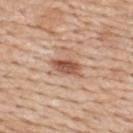Assessment:
Captured during whole-body skin photography for melanoma surveillance; the lesion was not biopsied.
Image and clinical context:
Located on the upper back. Longest diameter approximately 4 mm. A male patient in their 60s. A roughly 15 mm field-of-view crop from a total-body skin photograph.